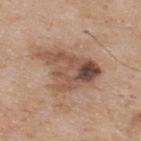Clinical impression: Recorded during total-body skin imaging; not selected for excision or biopsy. Clinical summary: A lesion tile, about 15 mm wide, cut from a 3D total-body photograph. Imaged with white-light lighting. The lesion is on the back. The patient is a male aged 73 to 77.